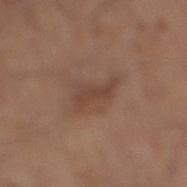Impression:
Part of a total-body skin-imaging series; this lesion was reviewed on a skin check and was not flagged for biopsy.
Context:
A male subject approximately 65 years of age. The tile uses white-light illumination. From the right lower leg. A 15 mm close-up tile from a total-body photography series done for melanoma screening. The lesion-visualizer software estimated a footprint of about 5.5 mm², an eccentricity of roughly 0.95, and a shape-asymmetry score of about 0.4 (0 = symmetric). It also reported a lesion color around L≈43 a*≈18 b*≈26 in CIELAB, roughly 7 lightness units darker than nearby skin, and a normalized border contrast of about 6.